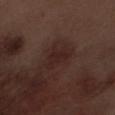biopsy status = catalogued during a skin exam; not biopsied
image source = ~15 mm crop, total-body skin-cancer survey
diameter = ≈3.5 mm
subject = male, approximately 70 years of age
site = the right forearm